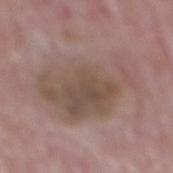follow-up: catalogued during a skin exam; not biopsied
size: ~9.5 mm (longest diameter)
acquisition: ~15 mm tile from a whole-body skin photo
anatomic site: the mid back
patient: male, aged 63 to 67
lighting: white-light